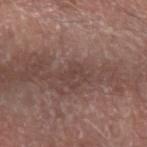| feature | finding |
|---|---|
| notes | catalogued during a skin exam; not biopsied |
| subject | male, roughly 65 years of age |
| anatomic site | the left forearm |
| image | ~15 mm tile from a whole-body skin photo |